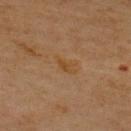The lesion was tiled from a total-body skin photograph and was not biopsied. The recorded lesion diameter is about 3 mm. A male patient, in their mid-60s. A 15 mm crop from a total-body photograph taken for skin-cancer surveillance. This is a cross-polarized tile. The lesion is on the upper back. Automated tile analysis of the lesion measured a lesion area of about 3 mm², a shape eccentricity near 0.95, and a symmetry-axis asymmetry near 0.4. The software also gave border irregularity of about 4.5 on a 0–10 scale and radial color variation of about 0. And it measured an automated nevus-likeness rating near 0 out of 100 and lesion-presence confidence of about 100/100.